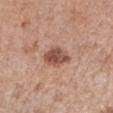biopsy status = catalogued during a skin exam; not biopsied
patient = female, approximately 60 years of age
body site = the arm
tile lighting = white-light illumination
image source = ~15 mm crop, total-body skin-cancer survey